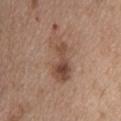Q: Was this lesion biopsied?
A: total-body-photography surveillance lesion; no biopsy
Q: What is the imaging modality?
A: 15 mm crop, total-body photography
Q: Lesion size?
A: about 6 mm
Q: Who is the patient?
A: female, aged around 45
Q: What lighting was used for the tile?
A: white-light illumination
Q: Lesion location?
A: the back